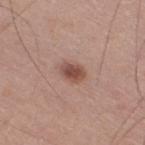Assessment: Imaged during a routine full-body skin examination; the lesion was not biopsied and no histopathology is available. Acquisition and patient details: Cropped from a whole-body photographic skin survey; the tile spans about 15 mm. Longest diameter approximately 3 mm. A male subject, aged 48 to 52. The lesion is located on the right thigh.